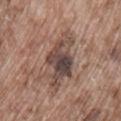Clinical impression:
Part of a total-body skin-imaging series; this lesion was reviewed on a skin check and was not flagged for biopsy.
Context:
A 15 mm crop from a total-body photograph taken for skin-cancer surveillance. A male patient, in their mid- to late 70s. The lesion is located on the lower back.